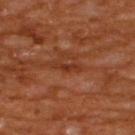Q: Was a biopsy performed?
A: catalogued during a skin exam; not biopsied
Q: Patient demographics?
A: male, about 65 years old
Q: Automated lesion metrics?
A: a lesion area of about 2 mm² and an outline eccentricity of about 0.95 (0 = round, 1 = elongated); a border-irregularity index near 5.5/10 and radial color variation of about 0; an automated nevus-likeness rating near 0 out of 100 and a lesion-detection confidence of about 90/100
Q: Lesion size?
A: about 2.5 mm
Q: What is the anatomic site?
A: the upper back
Q: How was this image acquired?
A: ~15 mm crop, total-body skin-cancer survey